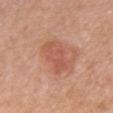– follow-up: imaged on a skin check; not biopsied
– diameter: about 4.5 mm
– subject: female, aged 53–57
– imaging modality: ~15 mm tile from a whole-body skin photo
– site: the chest
– illumination: white-light